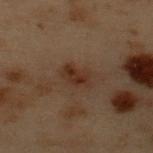| key | value |
|---|---|
| workup | catalogued during a skin exam; not biopsied |
| illumination | cross-polarized illumination |
| subject | male, aged approximately 55 |
| lesion size | ~3 mm (longest diameter) |
| body site | the upper back |
| automated lesion analysis | an area of roughly 5.5 mm², a shape eccentricity near 0.75, and a shape-asymmetry score of about 0.25 (0 = symmetric); an automated nevus-likeness rating near 15 out of 100 and a lesion-detection confidence of about 100/100 |
| imaging modality | ~15 mm tile from a whole-body skin photo |